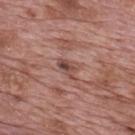{"site": "mid back", "image": {"source": "total-body photography crop", "field_of_view_mm": 15}, "patient": {"sex": "male", "age_approx": 70}}